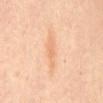Assessment: No biopsy was performed on this lesion — it was imaged during a full skin examination and was not determined to be concerning. Clinical summary: Cropped from a whole-body photographic skin survey; the tile spans about 15 mm. A female subject, approximately 45 years of age. Longest diameter approximately 4.5 mm. Automated image analysis of the tile measured a shape eccentricity near 0.95 and a symmetry-axis asymmetry near 0.25. The software also gave border irregularity of about 3.5 on a 0–10 scale and a color-variation rating of about 1.5/10. It also reported an automated nevus-likeness rating near 0 out of 100 and a detector confidence of about 100 out of 100 that the crop contains a lesion. Captured under cross-polarized illumination. Located on the back.